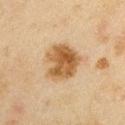<case>
  <biopsy_status>not biopsied; imaged during a skin examination</biopsy_status>
  <site>right upper arm</site>
  <patient>
    <sex>male</sex>
    <age_approx>45</age_approx>
  </patient>
  <lesion_size>
    <long_diameter_mm_approx>5.0</long_diameter_mm_approx>
  </lesion_size>
  <automated_metrics>
    <eccentricity>0.45</eccentricity>
    <shape_asymmetry>0.25</shape_asymmetry>
    <cielab_L>46</cielab_L>
    <cielab_a>16</cielab_a>
    <cielab_b>33</cielab_b>
    <vs_skin_darker_L>12.0</vs_skin_darker_L>
    <nevus_likeness_0_100>95</nevus_likeness_0_100>
    <lesion_detection_confidence_0_100>100</lesion_detection_confidence_0_100>
  </automated_metrics>
  <image>
    <source>total-body photography crop</source>
    <field_of_view_mm>15</field_of_view_mm>
  </image>
  <lighting>cross-polarized</lighting>
</case>